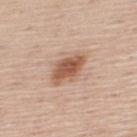Imaged during a routine full-body skin examination; the lesion was not biopsied and no histopathology is available. Cropped from a total-body skin-imaging series; the visible field is about 15 mm. Captured under white-light illumination. A male patient, aged 43 to 47. On the upper back. An algorithmic analysis of the crop reported border irregularity of about 2.5 on a 0–10 scale, a within-lesion color-variation index near 3.5/10, and radial color variation of about 1. And it measured a lesion-detection confidence of about 100/100. The lesion's longest dimension is about 4.5 mm.